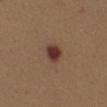Q: Is there a histopathology result?
A: catalogued during a skin exam; not biopsied
Q: How large is the lesion?
A: about 2.5 mm
Q: Lesion location?
A: the mid back
Q: What kind of image is this?
A: ~15 mm crop, total-body skin-cancer survey
Q: What are the patient's age and sex?
A: female, roughly 40 years of age
Q: What did automated image analysis measure?
A: about 13 CIELAB-L* units darker than the surrounding skin and a normalized lesion–skin contrast near 12.5; a lesion-detection confidence of about 100/100
Q: What lighting was used for the tile?
A: white-light illumination